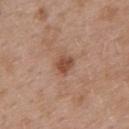follow-up: total-body-photography surveillance lesion; no biopsy | image source: 15 mm crop, total-body photography | tile lighting: white-light illumination | location: the upper back | patient: female, aged 38–42 | lesion size: about 2.5 mm | image-analysis metrics: a detector confidence of about 100 out of 100 that the crop contains a lesion.